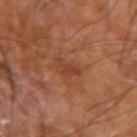biopsy status = no biopsy performed (imaged during a skin exam) | TBP lesion metrics = an area of roughly 3 mm², a shape eccentricity near 0.8, and a symmetry-axis asymmetry near 0.5; an average lesion color of about L≈40 a*≈26 b*≈32 (CIELAB) | tile lighting = cross-polarized | patient = aged approximately 65 | diameter = ~3 mm (longest diameter) | image = ~15 mm crop, total-body skin-cancer survey | anatomic site = the left forearm.